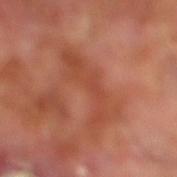Context: Automated tile analysis of the lesion measured a shape eccentricity near 0.9. The software also gave about 7 CIELAB-L* units darker than the surrounding skin and a lesion-to-skin contrast of about 5.5 (normalized; higher = more distinct). The software also gave a border-irregularity rating of about 8/10, internal color variation of about 3 on a 0–10 scale, and a peripheral color-asymmetry measure near 1. A 15 mm close-up tile from a total-body photography series done for melanoma screening. From the right lower leg. A male subject roughly 70 years of age. The tile uses cross-polarized illumination.